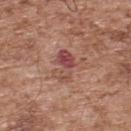The lesion was tiled from a total-body skin photograph and was not biopsied. The tile uses white-light illumination. A roughly 15 mm field-of-view crop from a total-body skin photograph. A male subject aged 53 to 57. From the upper back. An algorithmic analysis of the crop reported a nevus-likeness score of about 0/100 and lesion-presence confidence of about 100/100.